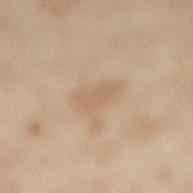TBP lesion metrics: an eccentricity of roughly 0.85 and a shape-asymmetry score of about 0.25 (0 = symmetric); anatomic site: the right lower leg; acquisition: ~15 mm crop, total-body skin-cancer survey; subject: female, roughly 35 years of age; diameter: ≈4 mm.